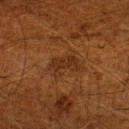| key | value |
|---|---|
| notes | catalogued during a skin exam; not biopsied |
| acquisition | ~15 mm tile from a whole-body skin photo |
| site | the right lower leg |
| subject | male, roughly 60 years of age |
| automated lesion analysis | a lesion area of about 6 mm², a shape eccentricity near 0.85, and two-axis asymmetry of about 0.35; an automated nevus-likeness rating near 0 out of 100 and lesion-presence confidence of about 95/100 |